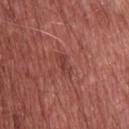follow-up=imaged on a skin check; not biopsied
lesion size=~3.5 mm (longest diameter)
anatomic site=the upper back
subject=male, aged 53–57
imaging modality=15 mm crop, total-body photography